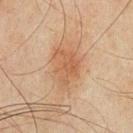Q: Was a biopsy performed?
A: no biopsy performed (imaged during a skin exam)
Q: Patient demographics?
A: male, roughly 45 years of age
Q: How was this image acquired?
A: ~15 mm crop, total-body skin-cancer survey
Q: What is the anatomic site?
A: the chest